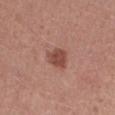The lesion was tiled from a total-body skin photograph and was not biopsied. Captured under white-light illumination. A close-up tile cropped from a whole-body skin photograph, about 15 mm across. A female patient, aged 23 to 27. Automated image analysis of the tile measured an average lesion color of about L≈47 a*≈24 b*≈26 (CIELAB), about 11 CIELAB-L* units darker than the surrounding skin, and a normalized border contrast of about 8.5. And it measured a border-irregularity rating of about 2/10, internal color variation of about 3 on a 0–10 scale, and peripheral color asymmetry of about 1. About 2.5 mm across. Located on the chest.